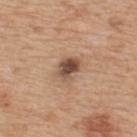Part of a total-body skin-imaging series; this lesion was reviewed on a skin check and was not flagged for biopsy. The tile uses white-light illumination. The lesion is on the upper back. The subject is a male approximately 65 years of age. Longest diameter approximately 3.5 mm. A lesion tile, about 15 mm wide, cut from a 3D total-body photograph.